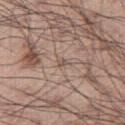biopsy status: imaged on a skin check; not biopsied
diameter: ~1 mm (longest diameter)
body site: the right thigh
image-analysis metrics: a shape eccentricity near 0.55 and two-axis asymmetry of about 0.45; about 8 CIELAB-L* units darker than the surrounding skin and a normalized lesion–skin contrast near 6; border irregularity of about 3 on a 0–10 scale, a color-variation rating of about 0/10, and radial color variation of about 0
patient: male, aged around 55
imaging modality: ~15 mm crop, total-body skin-cancer survey
lighting: white-light illumination